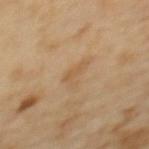Findings:
• notes: total-body-photography surveillance lesion; no biopsy
• lesion size: ~3 mm (longest diameter)
• site: the upper back
• subject: female, in their 60s
• image source: total-body-photography crop, ~15 mm field of view
• illumination: cross-polarized